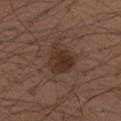Captured during whole-body skin photography for melanoma surveillance; the lesion was not biopsied.
Longest diameter approximately 3.5 mm.
The lesion-visualizer software estimated a lesion color around L≈31 a*≈17 b*≈23 in CIELAB, a lesion–skin lightness drop of about 8, and a normalized border contrast of about 8. The analysis additionally found a classifier nevus-likeness of about 80/100 and lesion-presence confidence of about 100/100.
A close-up tile cropped from a whole-body skin photograph, about 15 mm across.
The lesion is on the arm.
A male subject aged 38 to 42.